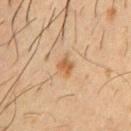This is a cross-polarized tile. The lesion-visualizer software estimated a lesion–skin lightness drop of about 9. And it measured border irregularity of about 3 on a 0–10 scale and a peripheral color-asymmetry measure near 0.5. The subject is a male aged approximately 60. The recorded lesion diameter is about 2.5 mm. A close-up tile cropped from a whole-body skin photograph, about 15 mm across. On the chest.